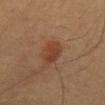The lesion was tiled from a total-body skin photograph and was not biopsied. Cropped from a whole-body photographic skin survey; the tile spans about 15 mm. The lesion's longest dimension is about 3.5 mm. A male subject about 60 years old. From the abdomen.